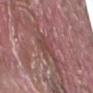This lesion was catalogued during total-body skin photography and was not selected for biopsy.
Captured under white-light illumination.
Approximately 3 mm at its widest.
A region of skin cropped from a whole-body photographic capture, roughly 15 mm wide.
The lesion is on the right forearm.
A male patient in their 40s.